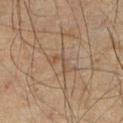{
  "biopsy_status": "not biopsied; imaged during a skin examination",
  "image": {
    "source": "total-body photography crop",
    "field_of_view_mm": 15
  },
  "lesion_size": {
    "long_diameter_mm_approx": 2.5
  },
  "automated_metrics": {
    "area_mm2_approx": 2.5,
    "eccentricity": 0.9,
    "shape_asymmetry": 0.6,
    "cielab_L": 49,
    "cielab_a": 16,
    "cielab_b": 29,
    "vs_skin_contrast_norm": 5.5
  },
  "site": "right lower leg",
  "lighting": "cross-polarized",
  "patient": {
    "sex": "male",
    "age_approx": 45
  }
}